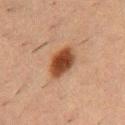Impression:
Imaged during a routine full-body skin examination; the lesion was not biopsied and no histopathology is available.
Clinical summary:
A male subject in their 50s. This is a cross-polarized tile. Automated image analysis of the tile measured an area of roughly 9 mm², an eccentricity of roughly 0.8, and two-axis asymmetry of about 0.15. The analysis additionally found a color-variation rating of about 4/10 and a peripheral color-asymmetry measure near 1. The lesion is located on the chest. A close-up tile cropped from a whole-body skin photograph, about 15 mm across.